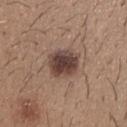Impression: No biopsy was performed on this lesion — it was imaged during a full skin examination and was not determined to be concerning. Clinical summary: The lesion is located on the upper back. Approximately 4 mm at its widest. A male patient, about 25 years old. A roughly 15 mm field-of-view crop from a total-body skin photograph. This is a white-light tile. Automated image analysis of the tile measured border irregularity of about 1.5 on a 0–10 scale, internal color variation of about 5 on a 0–10 scale, and peripheral color asymmetry of about 1.5. The analysis additionally found a nevus-likeness score of about 80/100 and lesion-presence confidence of about 100/100.